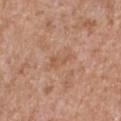workup: total-body-photography surveillance lesion; no biopsy | lighting: white-light illumination | body site: the chest | TBP lesion metrics: a shape-asymmetry score of about 0.45 (0 = symmetric); a mean CIELAB color near L≈55 a*≈22 b*≈32, about 6 CIELAB-L* units darker than the surrounding skin, and a lesion-to-skin contrast of about 5 (normalized; higher = more distinct); a border-irregularity rating of about 5/10 and a within-lesion color-variation index near 1/10; a classifier nevus-likeness of about 0/100 and a detector confidence of about 100 out of 100 that the crop contains a lesion | subject: male, approximately 65 years of age | image source: ~15 mm tile from a whole-body skin photo.